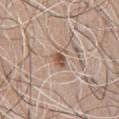Clinical impression:
The lesion was tiled from a total-body skin photograph and was not biopsied.
Acquisition and patient details:
The recorded lesion diameter is about 2.5 mm. A 15 mm close-up extracted from a 3D total-body photography capture. An algorithmic analysis of the crop reported an area of roughly 4 mm² and a symmetry-axis asymmetry near 0.35. It also reported about 11 CIELAB-L* units darker than the surrounding skin and a normalized lesion–skin contrast near 8. The analysis additionally found internal color variation of about 3.5 on a 0–10 scale and peripheral color asymmetry of about 1. The patient is a male aged around 65. The lesion is on the chest.